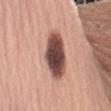Clinical impression:
The lesion was tiled from a total-body skin photograph and was not biopsied.
Acquisition and patient details:
Cropped from a total-body skin-imaging series; the visible field is about 15 mm. The lesion is on the left upper arm. A female subject, about 60 years old.